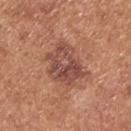– biopsy status — total-body-photography surveillance lesion; no biopsy
– lighting — white-light illumination
– image — total-body-photography crop, ~15 mm field of view
– body site — the back
– lesion size — about 5.5 mm
– patient — male, aged 63–67
– automated metrics — a nevus-likeness score of about 20/100